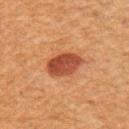The lesion was tiled from a total-body skin photograph and was not biopsied. The patient is a male approximately 50 years of age. Imaged with cross-polarized lighting. The total-body-photography lesion software estimated an area of roughly 9.5 mm², an outline eccentricity of about 0.65 (0 = round, 1 = elongated), and two-axis asymmetry of about 0.15. The software also gave an average lesion color of about L≈38 a*≈25 b*≈30 (CIELAB), a lesion–skin lightness drop of about 12, and a normalized lesion–skin contrast near 10. Cropped from a total-body skin-imaging series; the visible field is about 15 mm. Measured at roughly 4 mm in maximum diameter. The lesion is located on the right upper arm.